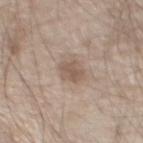Recorded during total-body skin imaging; not selected for excision or biopsy. The lesion is located on the chest. A male patient, aged around 80. A 15 mm close-up extracted from a 3D total-body photography capture. Imaged with white-light lighting. Longest diameter approximately 3 mm.